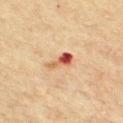Impression:
The lesion was photographed on a routine skin check and not biopsied; there is no pathology result.
Background:
From the chest. Cropped from a whole-body photographic skin survey; the tile spans about 15 mm. About 3.5 mm across. The subject is a male aged 58–62.